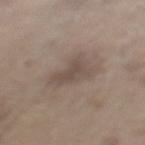A region of skin cropped from a whole-body photographic capture, roughly 15 mm wide.
The tile uses white-light illumination.
Located on the left lower leg.
The lesion-visualizer software estimated a lesion color around L≈49 a*≈12 b*≈21 in CIELAB, roughly 8 lightness units darker than nearby skin, and a normalized border contrast of about 6. The software also gave a border-irregularity index near 4.5/10.
A male subject aged around 70.
About 4.5 mm across.